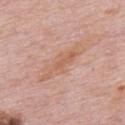| feature | finding |
|---|---|
| biopsy status | catalogued during a skin exam; not biopsied |
| imaging modality | ~15 mm crop, total-body skin-cancer survey |
| body site | the upper back |
| patient | male, aged 73 to 77 |